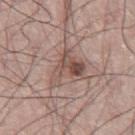Q: Is there a histopathology result?
A: no biopsy performed (imaged during a skin exam)
Q: How large is the lesion?
A: about 5 mm
Q: What is the imaging modality?
A: ~15 mm crop, total-body skin-cancer survey
Q: What lighting was used for the tile?
A: white-light illumination
Q: Who is the patient?
A: male, in their 70s
Q: Automated lesion metrics?
A: a footprint of about 9.5 mm² and an outline eccentricity of about 0.7 (0 = round, 1 = elongated); an average lesion color of about L≈50 a*≈18 b*≈23 (CIELAB), a lesion–skin lightness drop of about 10, and a normalized lesion–skin contrast near 7.5; a detector confidence of about 100 out of 100 that the crop contains a lesion
Q: Where on the body is the lesion?
A: the left leg